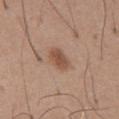Recorded during total-body skin imaging; not selected for excision or biopsy.
From the front of the torso.
Longest diameter approximately 3 mm.
Automated tile analysis of the lesion measured a lesion area of about 5 mm² and a shape eccentricity near 0.75. It also reported a color-variation rating of about 1.5/10 and radial color variation of about 0.5.
A male subject, aged approximately 65.
A close-up tile cropped from a whole-body skin photograph, about 15 mm across.